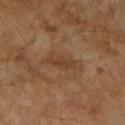{
  "biopsy_status": "not biopsied; imaged during a skin examination",
  "lesion_size": {
    "long_diameter_mm_approx": 3.5
  },
  "automated_metrics": {
    "area_mm2_approx": 4.5,
    "eccentricity": 0.95,
    "shape_asymmetry": 0.3,
    "cielab_L": 41,
    "cielab_a": 20,
    "cielab_b": 33,
    "vs_skin_darker_L": 7.0,
    "vs_skin_contrast_norm": 6.0,
    "nevus_likeness_0_100": 0
  },
  "lighting": "cross-polarized",
  "site": "left forearm",
  "image": {
    "source": "total-body photography crop",
    "field_of_view_mm": 15
  },
  "patient": {
    "sex": "female",
    "age_approx": 70
  }
}